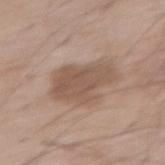This lesion was catalogued during total-body skin photography and was not selected for biopsy.
A male patient in their 70s.
On the right thigh.
A close-up tile cropped from a whole-body skin photograph, about 15 mm across.
The lesion-visualizer software estimated an outline eccentricity of about 0.75 (0 = round, 1 = elongated) and a symmetry-axis asymmetry near 0.25. And it measured a border-irregularity index near 3.5/10, a within-lesion color-variation index near 2.5/10, and radial color variation of about 1. It also reported an automated nevus-likeness rating near 25 out of 100 and a lesion-detection confidence of about 100/100.